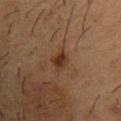notes: imaged on a skin check; not biopsied | image source: 15 mm crop, total-body photography | diameter: ~2.5 mm (longest diameter) | TBP lesion metrics: a footprint of about 4 mm²; a mean CIELAB color near L≈28 a*≈17 b*≈26, roughly 8 lightness units darker than nearby skin, and a lesion-to-skin contrast of about 9 (normalized; higher = more distinct); a detector confidence of about 100 out of 100 that the crop contains a lesion | tile lighting: cross-polarized | subject: male, in their mid-50s | body site: the front of the torso.